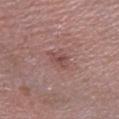Clinical impression:
No biopsy was performed on this lesion — it was imaged during a full skin examination and was not determined to be concerning.
Context:
This is a white-light tile. The lesion is on the left lower leg. Cropped from a total-body skin-imaging series; the visible field is about 15 mm. A male patient approximately 70 years of age. Longest diameter approximately 3 mm.